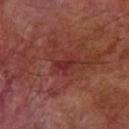The lesion was tiled from a total-body skin photograph and was not biopsied.
A 15 mm close-up extracted from a 3D total-body photography capture.
Imaged with cross-polarized lighting.
A male subject about 70 years old.
From the right forearm.
Approximately 2.5 mm at its widest.
An algorithmic analysis of the crop reported a mean CIELAB color near L≈30 a*≈30 b*≈23, roughly 6 lightness units darker than nearby skin, and a normalized border contrast of about 6.5. The software also gave a classifier nevus-likeness of about 0/100 and a lesion-detection confidence of about 90/100.